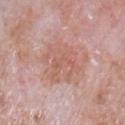workup=no biopsy performed (imaged during a skin exam); subject=male, aged 63 to 67; location=the front of the torso; diameter=about 6 mm; automated lesion analysis=a nevus-likeness score of about 0/100; acquisition=~15 mm tile from a whole-body skin photo.